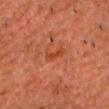{
  "biopsy_status": "not biopsied; imaged during a skin examination",
  "image": {
    "source": "total-body photography crop",
    "field_of_view_mm": 15
  },
  "site": "chest",
  "lighting": "cross-polarized",
  "patient": {
    "sex": "male",
    "age_approx": 80
  },
  "automated_metrics": {
    "nevus_likeness_0_100": 0,
    "lesion_detection_confidence_0_100": 100
  }
}